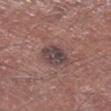Case summary:
* biopsy status — no biopsy performed (imaged during a skin exam)
* image — 15 mm crop, total-body photography
* illumination — white-light
* size — ~4.5 mm (longest diameter)
* patient — male, roughly 70 years of age
* body site — the leg